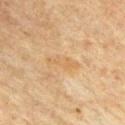Clinical impression: Part of a total-body skin-imaging series; this lesion was reviewed on a skin check and was not flagged for biopsy. Image and clinical context: A 15 mm crop from a total-body photograph taken for skin-cancer surveillance. Imaged with cross-polarized lighting. A male patient, aged approximately 65. From the chest. Approximately 4 mm at its widest.